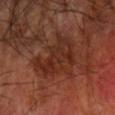Captured during whole-body skin photography for melanoma surveillance; the lesion was not biopsied.
The lesion is on the right forearm.
A roughly 15 mm field-of-view crop from a total-body skin photograph.
Automated tile analysis of the lesion measured a lesion area of about 20 mm². And it measured an average lesion color of about L≈29 a*≈23 b*≈27 (CIELAB), a lesion–skin lightness drop of about 7, and a normalized border contrast of about 6.5. The analysis additionally found a border-irregularity index near 7/10, internal color variation of about 5 on a 0–10 scale, and peripheral color asymmetry of about 1.5. And it measured a classifier nevus-likeness of about 0/100 and a lesion-detection confidence of about 95/100.
The patient is a male roughly 70 years of age.
The tile uses cross-polarized illumination.
The recorded lesion diameter is about 6 mm.